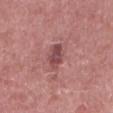biopsy_status: not biopsied; imaged during a skin examination
lesion_size:
  long_diameter_mm_approx: 3.5
patient:
  sex: male
  age_approx: 60
image:
  source: total-body photography crop
  field_of_view_mm: 15
lighting: white-light
site: back